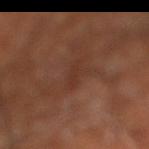Assessment:
Recorded during total-body skin imaging; not selected for excision or biopsy.
Image and clinical context:
From the right lower leg. Captured under cross-polarized illumination. A roughly 15 mm field-of-view crop from a total-body skin photograph. A subject aged 63–67.